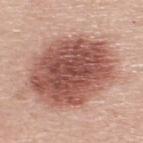Q: What lighting was used for the tile?
A: white-light
Q: Patient demographics?
A: male, aged 63 to 67
Q: Where on the body is the lesion?
A: the upper back
Q: What is the imaging modality?
A: ~15 mm crop, total-body skin-cancer survey
Q: Automated lesion metrics?
A: a lesion–skin lightness drop of about 17 and a lesion-to-skin contrast of about 11 (normalized; higher = more distinct); a detector confidence of about 100 out of 100 that the crop contains a lesion
Q: How large is the lesion?
A: about 9.5 mm